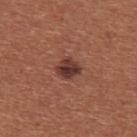  biopsy_status: not biopsied; imaged during a skin examination
  lighting: white-light
  patient:
    sex: female
    age_approx: 35
  image:
    source: total-body photography crop
    field_of_view_mm: 15
  lesion_size:
    long_diameter_mm_approx: 2.5
  site: chest